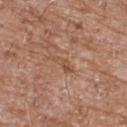Case summary:
- anatomic site — the chest
- size — ~3 mm (longest diameter)
- subject — male, aged 58 to 62
- image source — ~15 mm tile from a whole-body skin photo